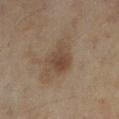Clinical impression: No biopsy was performed on this lesion — it was imaged during a full skin examination and was not determined to be concerning. Image and clinical context: A 15 mm crop from a total-body photograph taken for skin-cancer surveillance. Measured at roughly 3.5 mm in maximum diameter. This is a cross-polarized tile. A female subject aged 58–62. On the leg.